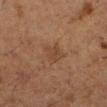The lesion was tiled from a total-body skin photograph and was not biopsied. A lesion tile, about 15 mm wide, cut from a 3D total-body photograph. A female subject, roughly 50 years of age. Captured under cross-polarized illumination. The lesion is on the leg. About 2.5 mm across.